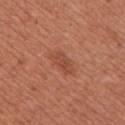workup: total-body-photography surveillance lesion; no biopsy
patient: female, roughly 25 years of age
lighting: white-light illumination
image-analysis metrics: an area of roughly 7 mm², an outline eccentricity of about 0.9 (0 = round, 1 = elongated), and a symmetry-axis asymmetry near 0.3
body site: the chest
image: 15 mm crop, total-body photography